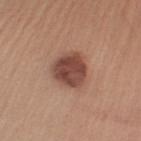Assessment:
Captured during whole-body skin photography for melanoma surveillance; the lesion was not biopsied.
Acquisition and patient details:
Automated tile analysis of the lesion measured a footprint of about 13 mm², an eccentricity of roughly 0.3, and two-axis asymmetry of about 0.2. A male subject, approximately 55 years of age. The lesion is on the left upper arm. This is a white-light tile. A 15 mm close-up extracted from a 3D total-body photography capture.